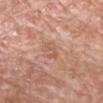Acquisition and patient details: Imaged with white-light lighting. A 15 mm close-up tile from a total-body photography series done for melanoma screening. From the head or neck. A male patient approximately 65 years of age. The total-body-photography lesion software estimated an area of roughly 3 mm², a shape eccentricity near 0.9, and a symmetry-axis asymmetry near 0.45. It also reported about 7 CIELAB-L* units darker than the surrounding skin and a lesion-to-skin contrast of about 5 (normalized; higher = more distinct). The software also gave a classifier nevus-likeness of about 0/100 and a lesion-detection confidence of about 100/100.